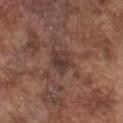Captured under white-light illumination.
The recorded lesion diameter is about 3.5 mm.
A male patient, approximately 75 years of age.
Cropped from a total-body skin-imaging series; the visible field is about 15 mm.
Located on the front of the torso.
The total-body-photography lesion software estimated a lesion area of about 6 mm², an outline eccentricity of about 0.65 (0 = round, 1 = elongated), and a shape-asymmetry score of about 0.35 (0 = symmetric). The software also gave a border-irregularity rating of about 3/10 and a color-variation rating of about 4/10.